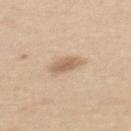Imaged during a routine full-body skin examination; the lesion was not biopsied and no histopathology is available. A female patient aged 38 to 42. About 3 mm across. On the upper back. A close-up tile cropped from a whole-body skin photograph, about 15 mm across.